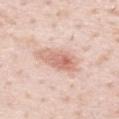A close-up tile cropped from a whole-body skin photograph, about 15 mm across.
Measured at roughly 4.5 mm in maximum diameter.
Automated image analysis of the tile measured a lesion area of about 10 mm² and an eccentricity of roughly 0.75. And it measured an average lesion color of about L≈68 a*≈22 b*≈28 (CIELAB) and a normalized border contrast of about 6.5.
On the upper back.
Imaged with white-light lighting.
A male patient, in their mid-30s.